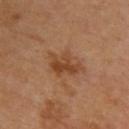Recorded during total-body skin imaging; not selected for excision or biopsy.